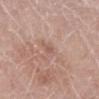No biopsy was performed on this lesion — it was imaged during a full skin examination and was not determined to be concerning.
This is a white-light tile.
A female patient, aged around 60.
About 1 mm across.
From the left lower leg.
A lesion tile, about 15 mm wide, cut from a 3D total-body photograph.
An algorithmic analysis of the crop reported a mean CIELAB color near L≈55 a*≈21 b*≈25 and a normalized lesion–skin contrast near 5. The software also gave a border-irregularity index near 2.5/10, a within-lesion color-variation index near 0/10, and peripheral color asymmetry of about 0. The software also gave a classifier nevus-likeness of about 0/100 and a lesion-detection confidence of about 100/100.